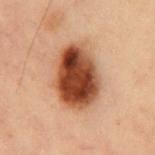Captured during whole-body skin photography for melanoma surveillance; the lesion was not biopsied. A male patient, in their mid-50s. Located on the chest. A 15 mm close-up extracted from a 3D total-body photography capture. Imaged with cross-polarized lighting.